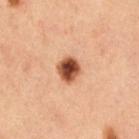biopsy status: total-body-photography surveillance lesion; no biopsy
imaging modality: ~15 mm tile from a whole-body skin photo
diameter: ≈3 mm
subject: male, approximately 60 years of age
automated lesion analysis: an area of roughly 6.5 mm², a shape eccentricity near 0.45, and a symmetry-axis asymmetry near 0.15; a lesion color around L≈38 a*≈21 b*≈28 in CIELAB, roughly 17 lightness units darker than nearby skin, and a normalized border contrast of about 13; border irregularity of about 1 on a 0–10 scale and peripheral color asymmetry of about 2; a nevus-likeness score of about 100/100
anatomic site: the left upper arm